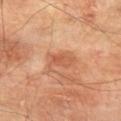Impression: No biopsy was performed on this lesion — it was imaged during a full skin examination and was not determined to be concerning.